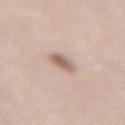Notes:
- follow-up · no biopsy performed (imaged during a skin exam)
- size · ≈2.5 mm
- lighting · white-light illumination
- body site · the lower back
- image · 15 mm crop, total-body photography
- patient · female, approximately 65 years of age
- TBP lesion metrics · an outline eccentricity of about 0.5 (0 = round, 1 = elongated) and a shape-asymmetry score of about 0.2 (0 = symmetric); an average lesion color of about L≈61 a*≈17 b*≈25 (CIELAB), a lesion–skin lightness drop of about 12, and a normalized border contrast of about 7.5